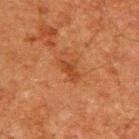Case summary:
• follow-up · imaged on a skin check; not biopsied
• anatomic site · the upper back
• imaging modality · ~15 mm tile from a whole-body skin photo
• patient · male, approximately 60 years of age